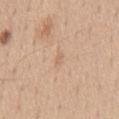Assessment:
Imaged during a routine full-body skin examination; the lesion was not biopsied and no histopathology is available.
Clinical summary:
A male subject aged around 55. On the front of the torso. A 15 mm crop from a total-body photograph taken for skin-cancer surveillance.